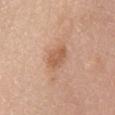Impression: Captured during whole-body skin photography for melanoma surveillance; the lesion was not biopsied. Image and clinical context: From the abdomen. The lesion's longest dimension is about 3.5 mm. An algorithmic analysis of the crop reported a footprint of about 7 mm² and a symmetry-axis asymmetry near 0.2. The software also gave an average lesion color of about L≈59 a*≈21 b*≈33 (CIELAB) and roughly 9 lightness units darker than nearby skin. It also reported border irregularity of about 2 on a 0–10 scale, a within-lesion color-variation index near 2.5/10, and radial color variation of about 1. The software also gave a nevus-likeness score of about 55/100 and lesion-presence confidence of about 100/100. A roughly 15 mm field-of-view crop from a total-body skin photograph. A female patient roughly 60 years of age. Captured under white-light illumination.